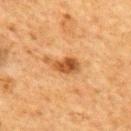  biopsy_status: not biopsied; imaged during a skin examination
  image:
    source: total-body photography crop
    field_of_view_mm: 15
  site: upper back
  lesion_size:
    long_diameter_mm_approx: 4.0
  patient:
    sex: female
    age_approx: 60
  lighting: cross-polarized